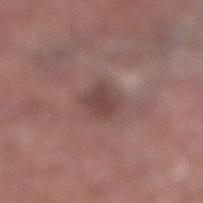  biopsy_status: not biopsied; imaged during a skin examination
  image:
    source: total-body photography crop
    field_of_view_mm: 15
  patient:
    sex: male
    age_approx: 55
  site: right lower leg
  lighting: white-light